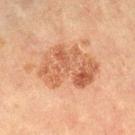  lesion_size:
    long_diameter_mm_approx: 7.5
  lighting: cross-polarized
  site: right lower leg
  automated_metrics:
    area_mm2_approx: 25.0
    eccentricity: 0.8
    shape_asymmetry: 0.25
    border_irregularity_0_10: 3.5
    color_variation_0_10: 5.5
    peripheral_color_asymmetry: 2.0
    lesion_detection_confidence_0_100: 100
  image:
    source: total-body photography crop
    field_of_view_mm: 15
  patient:
    sex: female
    age_approx: 60